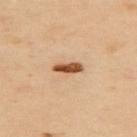A male subject, in their mid- to late 60s. From the upper back. The recorded lesion diameter is about 3.5 mm. Captured under cross-polarized illumination. The total-body-photography lesion software estimated an area of roughly 4.5 mm², an outline eccentricity of about 0.9 (0 = round, 1 = elongated), and a symmetry-axis asymmetry near 0.2. The software also gave a normalized lesion–skin contrast near 12. And it measured a border-irregularity rating of about 2/10 and a peripheral color-asymmetry measure near 1.5. A close-up tile cropped from a whole-body skin photograph, about 15 mm across.